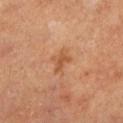notes: no biopsy performed (imaged during a skin exam); patient: male, aged 63 to 67; size: ~2.5 mm (longest diameter); image: 15 mm crop, total-body photography; location: the left lower leg; tile lighting: cross-polarized illumination.